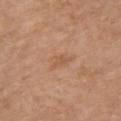| feature | finding |
|---|---|
| follow-up | catalogued during a skin exam; not biopsied |
| lesion size | about 3 mm |
| imaging modality | ~15 mm crop, total-body skin-cancer survey |
| body site | the front of the torso |
| patient | female, aged 53 to 57 |
| lighting | white-light |